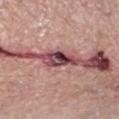workup — total-body-photography surveillance lesion; no biopsy | location — the left lower leg | lesion size — about 4.5 mm | image — ~15 mm crop, total-body skin-cancer survey | tile lighting — white-light illumination | patient — male, roughly 50 years of age.